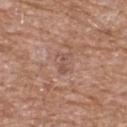{"biopsy_status": "not biopsied; imaged during a skin examination", "site": "chest", "lighting": "white-light", "image": {"source": "total-body photography crop", "field_of_view_mm": 15}, "lesion_size": {"long_diameter_mm_approx": 3.0}, "patient": {"sex": "male", "age_approx": 60}}